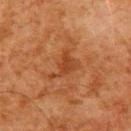Notes:
– workup: imaged on a skin check; not biopsied
– lesion diameter: ~4 mm (longest diameter)
– lighting: cross-polarized illumination
– location: the upper back
– acquisition: ~15 mm tile from a whole-body skin photo
– subject: male, roughly 80 years of age